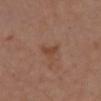Clinical impression: Imaged during a routine full-body skin examination; the lesion was not biopsied and no histopathology is available. Image and clinical context: A close-up tile cropped from a whole-body skin photograph, about 15 mm across. The total-body-photography lesion software estimated a border-irregularity index near 4.5/10 and internal color variation of about 1.5 on a 0–10 scale. The patient is a female about 40 years old. The tile uses white-light illumination. The lesion is on the chest.